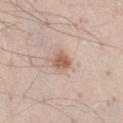| key | value |
|---|---|
| notes | total-body-photography surveillance lesion; no biopsy |
| site | the right thigh |
| TBP lesion metrics | a lesion area of about 5 mm², a shape eccentricity near 0.65, and two-axis asymmetry of about 0.2; border irregularity of about 2 on a 0–10 scale and a color-variation rating of about 3/10; a classifier nevus-likeness of about 90/100 and a lesion-detection confidence of about 100/100 |
| subject | male, about 40 years old |
| acquisition | ~15 mm crop, total-body skin-cancer survey |